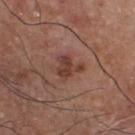location: the chest | subject: male, in their mid-70s | image: ~15 mm crop, total-body skin-cancer survey.